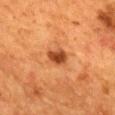Impression:
The lesion was tiled from a total-body skin photograph and was not biopsied.
Background:
A female patient, about 55 years old. Located on the mid back. Automated tile analysis of the lesion measured an area of roughly 5 mm², an outline eccentricity of about 0.6 (0 = round, 1 = elongated), and a shape-asymmetry score of about 0.2 (0 = symmetric). The analysis additionally found about 13 CIELAB-L* units darker than the surrounding skin and a lesion-to-skin contrast of about 10 (normalized; higher = more distinct). The analysis additionally found a nevus-likeness score of about 95/100 and a lesion-detection confidence of about 100/100. Captured under cross-polarized illumination. The recorded lesion diameter is about 2.5 mm. Cropped from a whole-body photographic skin survey; the tile spans about 15 mm.